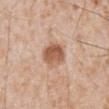A close-up tile cropped from a whole-body skin photograph, about 15 mm across. A male subject about 65 years old. Longest diameter approximately 3.5 mm. From the abdomen.